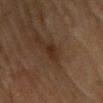The lesion was tiled from a total-body skin photograph and was not biopsied. The patient is a female roughly 80 years of age. From the chest. A close-up tile cropped from a whole-body skin photograph, about 15 mm across.